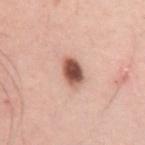| key | value |
|---|---|
| workup | imaged on a skin check; not biopsied |
| acquisition | 15 mm crop, total-body photography |
| site | the left upper arm |
| illumination | white-light illumination |
| size | ≈3 mm |
| TBP lesion metrics | a shape eccentricity near 0.65 and a shape-asymmetry score of about 0.2 (0 = symmetric); a lesion color around L≈54 a*≈24 b*≈27 in CIELAB and roughly 20 lightness units darker than nearby skin; a border-irregularity index near 2/10, a color-variation rating of about 5.5/10, and peripheral color asymmetry of about 1.5; a nevus-likeness score of about 100/100 and lesion-presence confidence of about 100/100 |
| patient | male, aged approximately 35 |